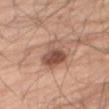Impression:
Recorded during total-body skin imaging; not selected for excision or biopsy.
Clinical summary:
This image is a 15 mm lesion crop taken from a total-body photograph. From the right thigh. The subject is a male aged 68–72. Measured at roughly 4.5 mm in maximum diameter.